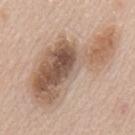Clinical impression: This lesion was catalogued during total-body skin photography and was not selected for biopsy.